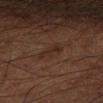Findings:
– follow-up: no biopsy performed (imaged during a skin exam)
– illumination: cross-polarized illumination
– lesion diameter: ~3 mm (longest diameter)
– imaging modality: 15 mm crop, total-body photography
– anatomic site: the left forearm
– subject: male, roughly 65 years of age